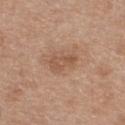{"biopsy_status": "not biopsied; imaged during a skin examination", "patient": {"sex": "female", "age_approx": 40}, "site": "back", "image": {"source": "total-body photography crop", "field_of_view_mm": 15}, "lighting": "white-light"}